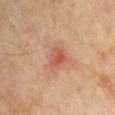The lesion's longest dimension is about 2.5 mm.
The lesion is on the left upper arm.
This is a cross-polarized tile.
A male patient aged 63–67.
A roughly 15 mm field-of-view crop from a total-body skin photograph.